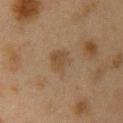Captured during whole-body skin photography for melanoma surveillance; the lesion was not biopsied. On the right upper arm. Approximately 3 mm at its widest. Cropped from a whole-body photographic skin survey; the tile spans about 15 mm. The tile uses cross-polarized illumination. The patient is a female in their 40s.